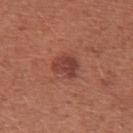Recorded during total-body skin imaging; not selected for excision or biopsy. A female subject approximately 35 years of age. The lesion is located on the left upper arm. A lesion tile, about 15 mm wide, cut from a 3D total-body photograph.